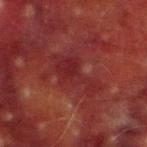Clinical impression:
The lesion was tiled from a total-body skin photograph and was not biopsied.
Context:
A male subject aged 68–72. A close-up tile cropped from a whole-body skin photograph, about 15 mm across. The tile uses cross-polarized illumination. Automated tile analysis of the lesion measured a lesion area of about 11 mm², an outline eccentricity of about 0.9 (0 = round, 1 = elongated), and a symmetry-axis asymmetry near 0.55. Approximately 6 mm at its widest. On the right lower leg.